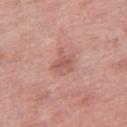Impression: Part of a total-body skin-imaging series; this lesion was reviewed on a skin check and was not flagged for biopsy. Clinical summary: Longest diameter approximately 3 mm. The lesion is on the right thigh. Imaged with white-light lighting. The total-body-photography lesion software estimated an outline eccentricity of about 0.45 (0 = round, 1 = elongated) and a shape-asymmetry score of about 0.4 (0 = symmetric). The analysis additionally found a lesion color around L≈57 a*≈24 b*≈26 in CIELAB, a lesion–skin lightness drop of about 8, and a normalized lesion–skin contrast near 5.5. And it measured border irregularity of about 4 on a 0–10 scale, internal color variation of about 3.5 on a 0–10 scale, and radial color variation of about 1. A female patient aged around 70. A lesion tile, about 15 mm wide, cut from a 3D total-body photograph.